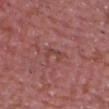workup — no biopsy performed (imaged during a skin exam)
acquisition — total-body-photography crop, ~15 mm field of view
automated metrics — a footprint of about 3.5 mm² and a shape eccentricity near 0.9; a color-variation rating of about 0/10 and radial color variation of about 0
tile lighting — white-light illumination
patient — male, in their mid-60s
anatomic site — the front of the torso
diameter — about 3.5 mm